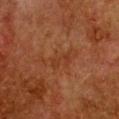Impression:
Part of a total-body skin-imaging series; this lesion was reviewed on a skin check and was not flagged for biopsy.
Image and clinical context:
A female subject, aged approximately 50. The lesion is located on the chest. A region of skin cropped from a whole-body photographic capture, roughly 15 mm wide.